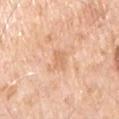Assessment:
The lesion was tiled from a total-body skin photograph and was not biopsied.
Clinical summary:
Located on the chest. A male subject, roughly 70 years of age. A roughly 15 mm field-of-view crop from a total-body skin photograph. The recorded lesion diameter is about 2.5 mm. Imaged with white-light lighting.